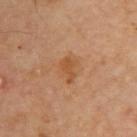Q: Is there a histopathology result?
A: imaged on a skin check; not biopsied
Q: What is the anatomic site?
A: the upper back
Q: What is the imaging modality?
A: total-body-photography crop, ~15 mm field of view
Q: What is the lesion's diameter?
A: ≈3 mm
Q: How was the tile lit?
A: cross-polarized illumination
Q: What are the patient's age and sex?
A: male, about 65 years old